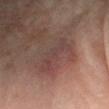Clinical impression: Part of a total-body skin-imaging series; this lesion was reviewed on a skin check and was not flagged for biopsy. Image and clinical context: A male subject, approximately 70 years of age. The lesion is located on the arm. A 15 mm close-up extracted from a 3D total-body photography capture.